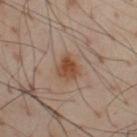Notes:
• workup: catalogued during a skin exam; not biopsied
• image: ~15 mm tile from a whole-body skin photo
• body site: the chest
• patient: male, aged approximately 55
• lighting: cross-polarized illumination
• lesion diameter: ~3 mm (longest diameter)